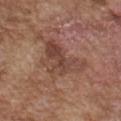follow-up = total-body-photography surveillance lesion; no biopsy | imaging modality = ~15 mm tile from a whole-body skin photo | automated lesion analysis = an area of roughly 17 mm² and an eccentricity of roughly 0.75; a normalized border contrast of about 6; a peripheral color-asymmetry measure near 2.5 | body site = the front of the torso | lighting = white-light illumination | subject = male, about 75 years old | size = ~6.5 mm (longest diameter).